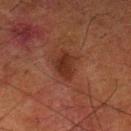Imaged during a routine full-body skin examination; the lesion was not biopsied and no histopathology is available.
Captured under cross-polarized illumination.
Automated image analysis of the tile measured an area of roughly 7 mm², an outline eccentricity of about 0.7 (0 = round, 1 = elongated), and a symmetry-axis asymmetry near 0.3. The software also gave border irregularity of about 3 on a 0–10 scale and a peripheral color-asymmetry measure near 0.5. The analysis additionally found a nevus-likeness score of about 30/100 and a lesion-detection confidence of about 100/100.
A male patient, about 80 years old.
A roughly 15 mm field-of-view crop from a total-body skin photograph.
Located on the leg.